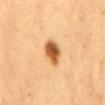This lesion was catalogued during total-body skin photography and was not selected for biopsy.